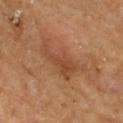  biopsy_status: not biopsied; imaged during a skin examination
  automated_metrics:
    area_mm2_approx: 13.0
    shape_asymmetry: 0.45
    vs_skin_darker_L: 8.0
    vs_skin_contrast_norm: 6.0
    color_variation_0_10: 3.5
    peripheral_color_asymmetry: 1.0
  patient:
    sex: female
    age_approx: 70
  site: upper back
  lighting: cross-polarized
  image:
    source: total-body photography crop
    field_of_view_mm: 15